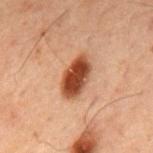Recorded during total-body skin imaging; not selected for excision or biopsy. A male patient aged 58 to 62. Imaged with cross-polarized lighting. A close-up tile cropped from a whole-body skin photograph, about 15 mm across. From the upper back. The lesion's longest dimension is about 5 mm.